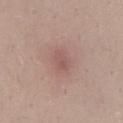<tbp_lesion>
  <biopsy_status>not biopsied; imaged during a skin examination</biopsy_status>
  <automated_metrics>
    <area_mm2_approx>5.0</area_mm2_approx>
    <eccentricity>0.75</eccentricity>
    <shape_asymmetry>0.2</shape_asymmetry>
    <cielab_L>54</cielab_L>
    <cielab_a>21</cielab_a>
    <cielab_b>23</cielab_b>
    <vs_skin_darker_L>7.0</vs_skin_darker_L>
    <nevus_likeness_0_100>10</nevus_likeness_0_100>
    <lesion_detection_confidence_0_100>100</lesion_detection_confidence_0_100>
  </automated_metrics>
  <image>
    <source>total-body photography crop</source>
    <field_of_view_mm>15</field_of_view_mm>
  </image>
  <lesion_size>
    <long_diameter_mm_approx>3.0</long_diameter_mm_approx>
  </lesion_size>
  <lighting>white-light</lighting>
  <site>lower back</site>
  <patient>
    <sex>male</sex>
    <age_approx>25</age_approx>
  </patient>
</tbp_lesion>